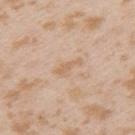Assessment:
The lesion was tiled from a total-body skin photograph and was not biopsied.
Acquisition and patient details:
The lesion-visualizer software estimated a normalized lesion–skin contrast near 5.5. A female subject aged around 25. The lesion is on the left upper arm. A lesion tile, about 15 mm wide, cut from a 3D total-body photograph. This is a white-light tile. Measured at roughly 3.5 mm in maximum diameter.